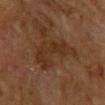notes=no biopsy performed (imaged during a skin exam) | tile lighting=cross-polarized | location=the chest | image source=~15 mm crop, total-body skin-cancer survey | size=~6.5 mm (longest diameter) | patient=female, roughly 80 years of age | automated metrics=an area of roughly 13 mm² and a symmetry-axis asymmetry near 0.45; a border-irregularity index near 7/10 and a color-variation rating of about 3/10.